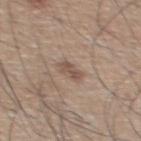| feature | finding |
|---|---|
| biopsy status | no biopsy performed (imaged during a skin exam) |
| imaging modality | 15 mm crop, total-body photography |
| patient | male, about 55 years old |
| body site | the upper back |